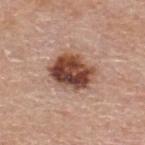workup: total-body-photography surveillance lesion; no biopsy | imaging modality: ~15 mm tile from a whole-body skin photo | patient: male, approximately 80 years of age | anatomic site: the upper back | size: about 5 mm | illumination: white-light | automated lesion analysis: a lesion area of about 15 mm² and a symmetry-axis asymmetry near 0.2; a mean CIELAB color near L≈45 a*≈23 b*≈28 and about 19 CIELAB-L* units darker than the surrounding skin; border irregularity of about 2 on a 0–10 scale and internal color variation of about 8.5 on a 0–10 scale.